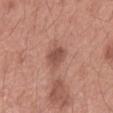biopsy_status: not biopsied; imaged during a skin examination
image:
  source: total-body photography crop
  field_of_view_mm: 15
patient:
  sex: female
  age_approx: 40
lesion_size:
  long_diameter_mm_approx: 2.5
lighting: white-light
site: right forearm
automated_metrics:
  area_mm2_approx: 5.0
  eccentricity: 0.45
  shape_asymmetry: 0.3
  nevus_likeness_0_100: 15